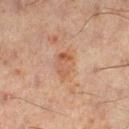Captured during whole-body skin photography for melanoma surveillance; the lesion was not biopsied. An algorithmic analysis of the crop reported a footprint of about 6 mm². It also reported an average lesion color of about L≈46 a*≈18 b*≈28 (CIELAB), about 6 CIELAB-L* units darker than the surrounding skin, and a normalized border contrast of about 6. It also reported a border-irregularity rating of about 2.5/10, internal color variation of about 4.5 on a 0–10 scale, and radial color variation of about 1.5. The patient is a male approximately 45 years of age. Located on the leg. Longest diameter approximately 3.5 mm. A 15 mm close-up extracted from a 3D total-body photography capture. Imaged with cross-polarized lighting.